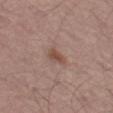patient=male, aged 53 to 57 | image=total-body-photography crop, ~15 mm field of view | location=the left thigh | automated lesion analysis=a lesion area of about 3 mm², an eccentricity of roughly 0.85, and a symmetry-axis asymmetry near 0.2; border irregularity of about 2 on a 0–10 scale and peripheral color asymmetry of about 0.5; an automated nevus-likeness rating near 55 out of 100 and lesion-presence confidence of about 100/100.